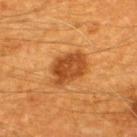Assessment: This lesion was catalogued during total-body skin photography and was not selected for biopsy. Acquisition and patient details: From the upper back. The patient is a male roughly 60 years of age. Automated image analysis of the tile measured a footprint of about 12 mm², a shape eccentricity near 0.7, and a shape-asymmetry score of about 0.25 (0 = symmetric). It also reported a normalized border contrast of about 8.5. The analysis additionally found border irregularity of about 2.5 on a 0–10 scale, a color-variation rating of about 3.5/10, and a peripheral color-asymmetry measure near 1. Cropped from a whole-body photographic skin survey; the tile spans about 15 mm. This is a cross-polarized tile. Measured at roughly 4.5 mm in maximum diameter.